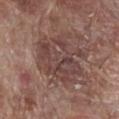biopsy_status: not biopsied; imaged during a skin examination
site: leg
patient:
  sex: male
  age_approx: 70
lighting: white-light
lesion_size:
  long_diameter_mm_approx: 6.5
automated_metrics:
  area_mm2_approx: 22.0
  eccentricity: 0.7
  shape_asymmetry: 0.25
  cielab_L: 42
  cielab_a: 18
  cielab_b: 21
  vs_skin_darker_L: 7.0
  vs_skin_contrast_norm: 6.0
  color_variation_0_10: 5.5
  peripheral_color_asymmetry: 2.0
  nevus_likeness_0_100: 0
  lesion_detection_confidence_0_100: 95
image:
  source: total-body photography crop
  field_of_view_mm: 15